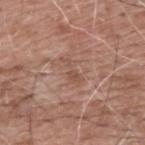biopsy_status: not biopsied; imaged during a skin examination
automated_metrics:
  area_mm2_approx: 3.5
  eccentricity: 0.95
  shape_asymmetry: 0.5
  border_irregularity_0_10: 6.5
  color_variation_0_10: 1.0
  peripheral_color_asymmetry: 0.0
lighting: white-light
site: upper back
image:
  source: total-body photography crop
  field_of_view_mm: 15
patient:
  sex: male
  age_approx: 60